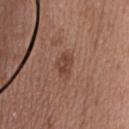Clinical impression:
This lesion was catalogued during total-body skin photography and was not selected for biopsy.
Acquisition and patient details:
Located on the upper back. A female patient roughly 50 years of age. Automated image analysis of the tile measured a border-irregularity index near 2.5/10, internal color variation of about 2.5 on a 0–10 scale, and radial color variation of about 1. The tile uses white-light illumination. The lesion's longest dimension is about 3 mm. A 15 mm close-up extracted from a 3D total-body photography capture.